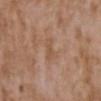follow-up = imaged on a skin check; not biopsied | lesion diameter = ~3 mm (longest diameter) | subject = female, roughly 35 years of age | body site = the upper back | imaging modality = total-body-photography crop, ~15 mm field of view | tile lighting = white-light illumination.